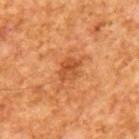Q: Is there a histopathology result?
A: imaged on a skin check; not biopsied